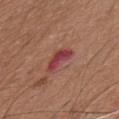Clinical impression:
Part of a total-body skin-imaging series; this lesion was reviewed on a skin check and was not flagged for biopsy.
Clinical summary:
Automated tile analysis of the lesion measured a footprint of about 5.5 mm², a shape eccentricity near 0.9, and a symmetry-axis asymmetry near 0.4. It also reported a border-irregularity rating of about 4.5/10, internal color variation of about 2.5 on a 0–10 scale, and a peripheral color-asymmetry measure near 0.5. The analysis additionally found a nevus-likeness score of about 0/100 and lesion-presence confidence of about 100/100. The lesion is on the chest. Imaged with white-light lighting. A male patient, in their 60s. Approximately 4 mm at its widest. A close-up tile cropped from a whole-body skin photograph, about 15 mm across.